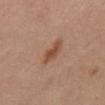Q: Is there a histopathology result?
A: total-body-photography surveillance lesion; no biopsy
Q: What lighting was used for the tile?
A: cross-polarized illumination
Q: What is the anatomic site?
A: the mid back
Q: What is the lesion's diameter?
A: ~3.5 mm (longest diameter)
Q: Who is the patient?
A: male, aged 58 to 62
Q: What is the imaging modality?
A: ~15 mm crop, total-body skin-cancer survey